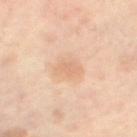Q: Was a biopsy performed?
A: imaged on a skin check; not biopsied
Q: How was this image acquired?
A: ~15 mm crop, total-body skin-cancer survey
Q: What lighting was used for the tile?
A: cross-polarized illumination
Q: How large is the lesion?
A: about 3 mm
Q: Lesion location?
A: the left thigh
Q: Who is the patient?
A: female, in their 50s
Q: What did automated image analysis measure?
A: an average lesion color of about L≈72 a*≈20 b*≈35 (CIELAB), a lesion–skin lightness drop of about 7, and a lesion-to-skin contrast of about 4.5 (normalized; higher = more distinct); a border-irregularity index near 3/10, a within-lesion color-variation index near 1/10, and a peripheral color-asymmetry measure near 0.5